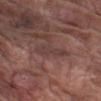{
  "biopsy_status": "not biopsied; imaged during a skin examination",
  "image": {
    "source": "total-body photography crop",
    "field_of_view_mm": 15
  },
  "lighting": "white-light",
  "patient": {
    "sex": "male",
    "age_approx": 75
  },
  "site": "left forearm",
  "lesion_size": {
    "long_diameter_mm_approx": 4.0
  }
}